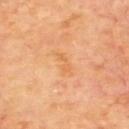Clinical impression: Captured during whole-body skin photography for melanoma surveillance; the lesion was not biopsied. Clinical summary: From the upper back. About 3 mm across. Captured under cross-polarized illumination. Cropped from a total-body skin-imaging series; the visible field is about 15 mm. A male subject, roughly 60 years of age.